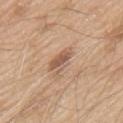• notes — imaged on a skin check; not biopsied
• image — ~15 mm tile from a whole-body skin photo
• subject — male, aged approximately 80
• body site — the upper back